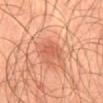| key | value |
|---|---|
| workup | total-body-photography surveillance lesion; no biopsy |
| lesion diameter | ≈3.5 mm |
| patient | male, aged approximately 45 |
| tile lighting | cross-polarized illumination |
| image source | ~15 mm tile from a whole-body skin photo |
| location | the mid back |